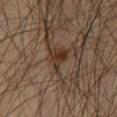Q: Is there a histopathology result?
A: catalogued during a skin exam; not biopsied
Q: How large is the lesion?
A: ~3.5 mm (longest diameter)
Q: Lesion location?
A: the left thigh
Q: What lighting was used for the tile?
A: cross-polarized illumination
Q: Who is the patient?
A: male, about 45 years old
Q: What kind of image is this?
A: ~15 mm tile from a whole-body skin photo